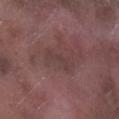Recorded during total-body skin imaging; not selected for excision or biopsy. The lesion-visualizer software estimated a lesion color around L≈38 a*≈18 b*≈17 in CIELAB, about 5 CIELAB-L* units darker than the surrounding skin, and a lesion-to-skin contrast of about 4.5 (normalized; higher = more distinct). The software also gave a classifier nevus-likeness of about 0/100. Located on the right lower leg. A 15 mm close-up tile from a total-body photography series done for melanoma screening. Approximately 4 mm at its widest. A male patient aged 73 to 77.